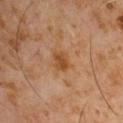biopsy status — imaged on a skin check; not biopsied | location — the chest | illumination — cross-polarized | size — ≈2.5 mm | acquisition — total-body-photography crop, ~15 mm field of view | patient — male, aged 58 to 62.